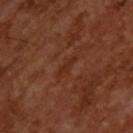{"biopsy_status": "not biopsied; imaged during a skin examination", "image": {"source": "total-body photography crop", "field_of_view_mm": 15}, "patient": {"sex": "male", "age_approx": 65}, "lesion_size": {"long_diameter_mm_approx": 3.0}, "automated_metrics": {"eccentricity": 0.95, "cielab_L": 28, "cielab_a": 23, "cielab_b": 29, "nevus_likeness_0_100": 0}}